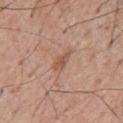This lesion was catalogued during total-body skin photography and was not selected for biopsy.
On the upper back.
About 3 mm across.
A male subject, aged approximately 65.
A roughly 15 mm field-of-view crop from a total-body skin photograph.